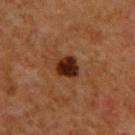biopsy_status: not biopsied; imaged during a skin examination